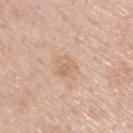<tbp_lesion>
  <biopsy_status>not biopsied; imaged during a skin examination</biopsy_status>
  <automated_metrics>
    <area_mm2_approx>3.5</area_mm2_approx>
    <eccentricity>0.7</eccentricity>
    <border_irregularity_0_10>3.5</border_irregularity_0_10>
    <color_variation_0_10>1.5</color_variation_0_10>
  </automated_metrics>
  <image>
    <source>total-body photography crop</source>
    <field_of_view_mm>15</field_of_view_mm>
  </image>
  <lesion_size>
    <long_diameter_mm_approx>2.5</long_diameter_mm_approx>
  </lesion_size>
  <lighting>white-light</lighting>
  <site>back</site>
  <patient>
    <sex>male</sex>
    <age_approx>60</age_approx>
  </patient>
</tbp_lesion>